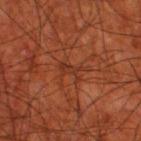Assessment:
No biopsy was performed on this lesion — it was imaged during a full skin examination and was not determined to be concerning.
Acquisition and patient details:
Captured under cross-polarized illumination. A 15 mm close-up extracted from a 3D total-body photography capture. On the right thigh. The patient is a male aged 68 to 72. Automated tile analysis of the lesion measured an area of roughly 3 mm² and a shape-asymmetry score of about 0.5 (0 = symmetric).